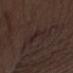No biopsy was performed on this lesion — it was imaged during a full skin examination and was not determined to be concerning.
The lesion-visualizer software estimated a lesion area of about 12 mm², a shape eccentricity near 0.6, and a shape-asymmetry score of about 0.4 (0 = symmetric). The software also gave a border-irregularity rating of about 6.5/10 and peripheral color asymmetry of about 1. The analysis additionally found an automated nevus-likeness rating near 0 out of 100 and a lesion-detection confidence of about 85/100.
The patient is a male aged approximately 70.
Measured at roughly 5 mm in maximum diameter.
This image is a 15 mm lesion crop taken from a total-body photograph.
This is a white-light tile.
The lesion is on the right upper arm.